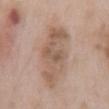Case summary:
• follow-up: no biopsy performed (imaged during a skin exam)
• image source: total-body-photography crop, ~15 mm field of view
• image-analysis metrics: lesion-presence confidence of about 100/100
• subject: male, aged 63–67
• body site: the mid back
• illumination: white-light illumination
• size: ~8.5 mm (longest diameter)